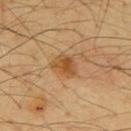Automated tile analysis of the lesion measured an area of roughly 6.5 mm² and an eccentricity of roughly 0.65. The analysis additionally found a border-irregularity index near 2/10, a within-lesion color-variation index near 4.5/10, and radial color variation of about 1.5. The software also gave an automated nevus-likeness rating near 60 out of 100 and a lesion-detection confidence of about 100/100. Cropped from a total-body skin-imaging series; the visible field is about 15 mm. The lesion is located on the upper back. About 3.5 mm across. A male patient, approximately 65 years of age.